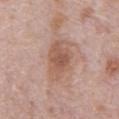| feature | finding |
|---|---|
| follow-up | catalogued during a skin exam; not biopsied |
| image | ~15 mm tile from a whole-body skin photo |
| patient | male, approximately 70 years of age |
| location | the abdomen |
| lighting | white-light illumination |